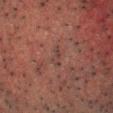Recorded during total-body skin imaging; not selected for excision or biopsy. Automated tile analysis of the lesion measured a lesion area of about 2.5 mm², a shape eccentricity near 0.9, and two-axis asymmetry of about 0.45. A male subject aged around 50. Captured under cross-polarized illumination. The lesion is located on the chest. A 15 mm crop from a total-body photograph taken for skin-cancer surveillance.